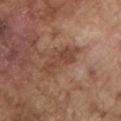Image and clinical context: The tile uses white-light illumination. Cropped from a whole-body photographic skin survey; the tile spans about 15 mm. Located on the left upper arm. A male subject, approximately 75 years of age. The total-body-photography lesion software estimated a mean CIELAB color near L≈44 a*≈21 b*≈28, about 9 CIELAB-L* units darker than the surrounding skin, and a normalized border contrast of about 6.5. And it measured a color-variation rating of about 2/10 and peripheral color asymmetry of about 0.5. About 5 mm across.